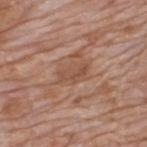  biopsy_status: not biopsied; imaged during a skin examination
  lesion_size:
    long_diameter_mm_approx: 3.5
  site: upper back
  lighting: white-light
  automated_metrics:
    area_mm2_approx: 5.0
    eccentricity: 0.85
    shape_asymmetry: 0.35
    border_irregularity_0_10: 4.0
    color_variation_0_10: 2.5
    peripheral_color_asymmetry: 1.0
  patient:
    sex: male
    age_approx: 60
  image:
    source: total-body photography crop
    field_of_view_mm: 15